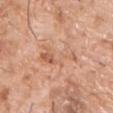This lesion was catalogued during total-body skin photography and was not selected for biopsy. A roughly 15 mm field-of-view crop from a total-body skin photograph. A male subject, aged 73–77. From the chest.